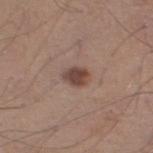Part of a total-body skin-imaging series; this lesion was reviewed on a skin check and was not flagged for biopsy.
Cropped from a total-body skin-imaging series; the visible field is about 15 mm.
This is a white-light tile.
The subject is a male about 40 years old.
An algorithmic analysis of the crop reported a lesion area of about 4.5 mm², a shape eccentricity near 0.7, and a symmetry-axis asymmetry near 0.15. And it measured a lesion color around L≈44 a*≈18 b*≈24 in CIELAB, a lesion–skin lightness drop of about 12, and a normalized border contrast of about 9.5. And it measured an automated nevus-likeness rating near 85 out of 100 and a detector confidence of about 100 out of 100 that the crop contains a lesion.
Measured at roughly 3 mm in maximum diameter.
From the right lower leg.